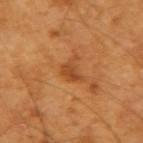biopsy status=total-body-photography surveillance lesion; no biopsy
illumination=cross-polarized
body site=the left forearm
TBP lesion metrics=border irregularity of about 5 on a 0–10 scale, a within-lesion color-variation index near 2.5/10, and radial color variation of about 1; a classifier nevus-likeness of about 30/100 and a lesion-detection confidence of about 100/100
patient=male, about 60 years old
image source=~15 mm tile from a whole-body skin photo
lesion diameter=~3 mm (longest diameter)